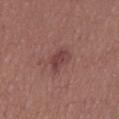Findings:
• workup · no biopsy performed (imaged during a skin exam)
• lighting · white-light illumination
• image source · ~15 mm crop, total-body skin-cancer survey
• subject · female, in their 40s
• diameter · ≈3.5 mm
• body site · the left thigh